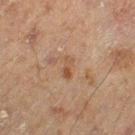Captured during whole-body skin photography for melanoma surveillance; the lesion was not biopsied.
Captured under cross-polarized illumination.
Longest diameter approximately 2.5 mm.
A male subject, aged approximately 70.
Cropped from a whole-body photographic skin survey; the tile spans about 15 mm.
From the left thigh.